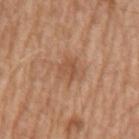Part of a total-body skin-imaging series; this lesion was reviewed on a skin check and was not flagged for biopsy. The tile uses white-light illumination. Located on the arm. A region of skin cropped from a whole-body photographic capture, roughly 15 mm wide. A male subject aged 63 to 67.